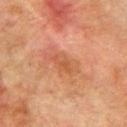Q: Is there a histopathology result?
A: imaged on a skin check; not biopsied
Q: What lighting was used for the tile?
A: cross-polarized
Q: What is the imaging modality?
A: ~15 mm tile from a whole-body skin photo
Q: Patient demographics?
A: male, approximately 75 years of age
Q: What did automated image analysis measure?
A: an area of roughly 4 mm², an eccentricity of roughly 0.8, and a shape-asymmetry score of about 0.3 (0 = symmetric); an average lesion color of about L≈45 a*≈23 b*≈32 (CIELAB), roughly 6 lightness units darker than nearby skin, and a normalized lesion–skin contrast near 5
Q: Where on the body is the lesion?
A: the arm